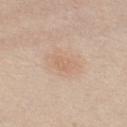Imaged during a routine full-body skin examination; the lesion was not biopsied and no histopathology is available.
A female subject aged 18 to 22.
This image is a 15 mm lesion crop taken from a total-body photograph.
On the chest.
The tile uses white-light illumination.